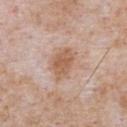Clinical impression:
Imaged during a routine full-body skin examination; the lesion was not biopsied and no histopathology is available.
Background:
The subject is a male aged 63–67. The tile uses white-light illumination. The lesion-visualizer software estimated an average lesion color of about L≈59 a*≈20 b*≈31 (CIELAB) and a lesion–skin lightness drop of about 10. The recorded lesion diameter is about 4 mm. A lesion tile, about 15 mm wide, cut from a 3D total-body photograph. The lesion is located on the chest.